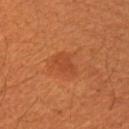  biopsy_status: not biopsied; imaged during a skin examination
  patient:
    sex: male
    age_approx: 60
  image:
    source: total-body photography crop
    field_of_view_mm: 15
  lesion_size:
    long_diameter_mm_approx: 3.0
  lighting: cross-polarized
  site: right upper arm